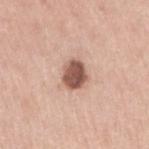<tbp_lesion>
<site>arm</site>
<automated_metrics>
  <area_mm2_approx>8.0</area_mm2_approx>
  <eccentricity>0.6</eccentricity>
  <shape_asymmetry>0.2</shape_asymmetry>
  <cielab_L>55</cielab_L>
  <cielab_a>21</cielab_a>
  <cielab_b>26</cielab_b>
  <vs_skin_contrast_norm>11.0</vs_skin_contrast_norm>
</automated_metrics>
<image>
  <source>total-body photography crop</source>
  <field_of_view_mm>15</field_of_view_mm>
</image>
<patient>
  <sex>female</sex>
  <age_approx>50</age_approx>
</patient>
<lighting>white-light</lighting>
<diagnosis>
  <histopathology>dysplastic (Clark) nevus</histopathology>
  <malignancy>benign</malignancy>
  <taxonomic_path>Benign; Benign melanocytic proliferations; Nevus; Nevus, Atypical, Dysplastic, or Clark</taxonomic_path>
</diagnosis>
</tbp_lesion>